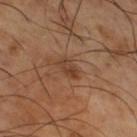biopsy status = imaged on a skin check; not biopsied | body site = the right thigh | lesion diameter = about 3 mm | patient = male, about 65 years old | tile lighting = cross-polarized | image = 15 mm crop, total-body photography.